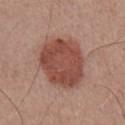Recorded during total-body skin imaging; not selected for excision or biopsy. The recorded lesion diameter is about 6.5 mm. Captured under white-light illumination. The lesion is located on the chest. A 15 mm close-up tile from a total-body photography series done for melanoma screening. A male subject, aged 23–27.